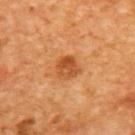Part of a total-body skin-imaging series; this lesion was reviewed on a skin check and was not flagged for biopsy.
The recorded lesion diameter is about 3 mm.
A roughly 15 mm field-of-view crop from a total-body skin photograph.
This is a cross-polarized tile.
A female subject roughly 40 years of age.
From the upper back.